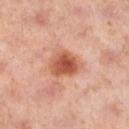Findings:
- follow-up: imaged on a skin check; not biopsied
- tile lighting: cross-polarized illumination
- lesion size: ~4 mm (longest diameter)
- subject: female, aged 38 to 42
- body site: the left lower leg
- acquisition: total-body-photography crop, ~15 mm field of view